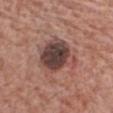image-analysis metrics: an area of roughly 17 mm² and an eccentricity of roughly 0.4; a border-irregularity rating of about 3/10, internal color variation of about 7 on a 0–10 scale, and a peripheral color-asymmetry measure near 1.5
acquisition: total-body-photography crop, ~15 mm field of view
body site: the chest
size: about 5 mm
subject: male, aged approximately 60
illumination: white-light